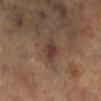biopsy_status: not biopsied; imaged during a skin examination
lighting: cross-polarized
patient:
  sex: female
  age_approx: 65
image:
  source: total-body photography crop
  field_of_view_mm: 15
lesion_size:
  long_diameter_mm_approx: 2.5
site: left leg